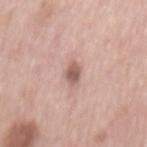Impression: Captured during whole-body skin photography for melanoma surveillance; the lesion was not biopsied. Acquisition and patient details: The patient is a male roughly 65 years of age. From the mid back. About 2.5 mm across. A region of skin cropped from a whole-body photographic capture, roughly 15 mm wide. The tile uses white-light illumination.